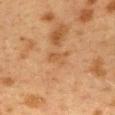Q: Was this lesion biopsied?
A: catalogued during a skin exam; not biopsied
Q: What is the lesion's diameter?
A: ≈2.5 mm
Q: Patient demographics?
A: female, aged around 40
Q: What is the anatomic site?
A: the mid back
Q: How was the tile lit?
A: cross-polarized illumination
Q: How was this image acquired?
A: ~15 mm tile from a whole-body skin photo